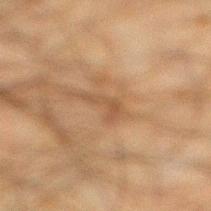  biopsy_status: not biopsied; imaged during a skin examination
  image:
    source: total-body photography crop
    field_of_view_mm: 15
  patient:
    sex: male
    age_approx: 35
  site: right lower leg